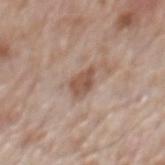Captured during whole-body skin photography for melanoma surveillance; the lesion was not biopsied. A 15 mm close-up extracted from a 3D total-body photography capture. Captured under white-light illumination. The patient is a male aged 68 to 72. The lesion is on the mid back. About 3 mm across.